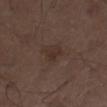Assessment: Recorded during total-body skin imaging; not selected for excision or biopsy. Acquisition and patient details: Measured at roughly 2.5 mm in maximum diameter. A region of skin cropped from a whole-body photographic capture, roughly 15 mm wide. A male patient, aged 48–52. The lesion is on the leg. An algorithmic analysis of the crop reported a mean CIELAB color near L≈30 a*≈14 b*≈21, roughly 5 lightness units darker than nearby skin, and a normalized lesion–skin contrast near 5.5. Imaged with white-light lighting.